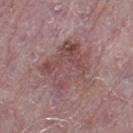Assessment:
Part of a total-body skin-imaging series; this lesion was reviewed on a skin check and was not flagged for biopsy.
Acquisition and patient details:
Longest diameter approximately 6 mm. The lesion is located on the right lower leg. A 15 mm crop from a total-body photograph taken for skin-cancer surveillance. A male subject, roughly 75 years of age. The tile uses white-light illumination.